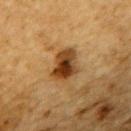Assessment:
Captured during whole-body skin photography for melanoma surveillance; the lesion was not biopsied.
Acquisition and patient details:
About 4 mm across. A male patient in their mid- to late 80s or older. On the upper back. The tile uses cross-polarized illumination. Automated image analysis of the tile measured a nevus-likeness score of about 95/100 and a lesion-detection confidence of about 100/100. A lesion tile, about 15 mm wide, cut from a 3D total-body photograph.